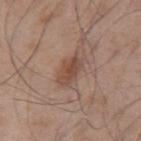Assessment: Imaged during a routine full-body skin examination; the lesion was not biopsied and no histopathology is available. Acquisition and patient details: The tile uses white-light illumination. The total-body-photography lesion software estimated an area of roughly 6 mm², an eccentricity of roughly 0.85, and a shape-asymmetry score of about 0.2 (0 = symmetric). The analysis additionally found a lesion color around L≈47 a*≈19 b*≈27 in CIELAB and a normalized lesion–skin contrast near 7.5. The lesion is located on the mid back. About 3.5 mm across. A male subject, in their mid- to late 50s. A lesion tile, about 15 mm wide, cut from a 3D total-body photograph.